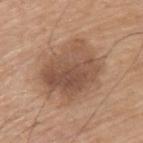notes=catalogued during a skin exam; not biopsied
location=the upper back
image source=~15 mm crop, total-body skin-cancer survey
patient=male, roughly 75 years of age